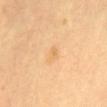{
  "biopsy_status": "not biopsied; imaged during a skin examination",
  "lesion_size": {
    "long_diameter_mm_approx": 1.5
  },
  "patient": {
    "sex": "male",
    "age_approx": 60
  },
  "lighting": "cross-polarized",
  "site": "abdomen",
  "image": {
    "source": "total-body photography crop",
    "field_of_view_mm": 15
  }
}